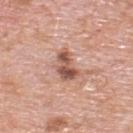Part of a total-body skin-imaging series; this lesion was reviewed on a skin check and was not flagged for biopsy.
A lesion tile, about 15 mm wide, cut from a 3D total-body photograph.
Located on the upper back.
A male subject roughly 80 years of age.